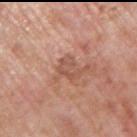Q: Was this lesion biopsied?
A: catalogued during a skin exam; not biopsied
Q: What did automated image analysis measure?
A: border irregularity of about 6 on a 0–10 scale, a color-variation rating of about 1.5/10, and radial color variation of about 0.5
Q: Where on the body is the lesion?
A: the arm
Q: Who is the patient?
A: male, aged around 70
Q: How large is the lesion?
A: about 3 mm
Q: What kind of image is this?
A: 15 mm crop, total-body photography